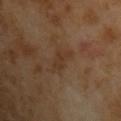Case summary:
* workup · catalogued during a skin exam; not biopsied
* tile lighting · cross-polarized illumination
* diameter · about 4.5 mm
* subject · male, in their mid-60s
* acquisition · 15 mm crop, total-body photography